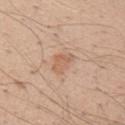A male subject roughly 30 years of age. A region of skin cropped from a whole-body photographic capture, roughly 15 mm wide. Located on the right upper arm.